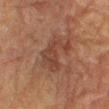Notes:
– patient · male, aged 83 or older
– acquisition · total-body-photography crop, ~15 mm field of view
– illumination · cross-polarized
– lesion diameter · ~5.5 mm (longest diameter)
– body site · the right thigh